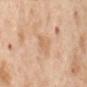Clinical summary:
The lesion's longest dimension is about 3 mm. Captured under cross-polarized illumination. The lesion is on the mid back. A 15 mm close-up extracted from a 3D total-body photography capture. A female patient, roughly 55 years of age.